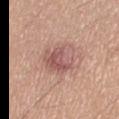{
  "biopsy_status": "not biopsied; imaged during a skin examination",
  "lesion_size": {
    "long_diameter_mm_approx": 5.5
  },
  "site": "right thigh",
  "patient": {
    "sex": "female",
    "age_approx": 45
  },
  "lighting": "white-light",
  "image": {
    "source": "total-body photography crop",
    "field_of_view_mm": 15
  }
}